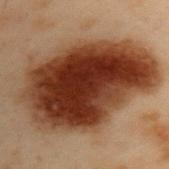lighting: cross-polarized
automated lesion analysis: a lesion area of about 80 mm², an outline eccentricity of about 0.8 (0 = round, 1 = elongated), and two-axis asymmetry of about 0.2; a border-irregularity rating of about 2.5/10 and radial color variation of about 3; a nevus-likeness score of about 100/100 and lesion-presence confidence of about 100/100
patient: male, aged 53–57
imaging modality: ~15 mm tile from a whole-body skin photo
size: ≈13 mm
anatomic site: the upper back